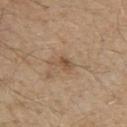Q: Is there a histopathology result?
A: no biopsy performed (imaged during a skin exam)
Q: What lighting was used for the tile?
A: white-light illumination
Q: How large is the lesion?
A: ≈3 mm
Q: Who is the patient?
A: male, aged around 70
Q: What kind of image is this?
A: ~15 mm tile from a whole-body skin photo
Q: What is the anatomic site?
A: the chest